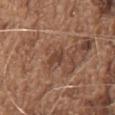biopsy status=no biopsy performed (imaged during a skin exam)
subject=male, aged 73–77
tile lighting=white-light illumination
imaging modality=15 mm crop, total-body photography
location=the front of the torso